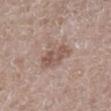follow-up: imaged on a skin check; not biopsied
imaging modality: total-body-photography crop, ~15 mm field of view
lighting: white-light
lesion diameter: about 4 mm
anatomic site: the right lower leg
patient: male, roughly 50 years of age